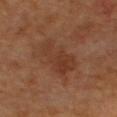patient: male, aged 58 to 62 | location: the upper back | acquisition: total-body-photography crop, ~15 mm field of view | TBP lesion metrics: an outline eccentricity of about 0.85 (0 = round, 1 = elongated); an automated nevus-likeness rating near 0 out of 100 and lesion-presence confidence of about 100/100.